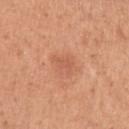The lesion was tiled from a total-body skin photograph and was not biopsied. The subject is a female approximately 55 years of age. On the left upper arm. Longest diameter approximately 2.5 mm. This image is a 15 mm lesion crop taken from a total-body photograph.